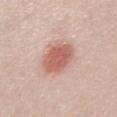Q: Was this lesion biopsied?
A: catalogued during a skin exam; not biopsied
Q: What is the imaging modality?
A: ~15 mm crop, total-body skin-cancer survey
Q: What did automated image analysis measure?
A: an area of roughly 11 mm² and a shape-asymmetry score of about 0.1 (0 = symmetric); roughly 13 lightness units darker than nearby skin and a lesion-to-skin contrast of about 8.5 (normalized; higher = more distinct); a border-irregularity index near 1.5/10, internal color variation of about 3 on a 0–10 scale, and radial color variation of about 1; an automated nevus-likeness rating near 100 out of 100 and lesion-presence confidence of about 100/100
Q: Where on the body is the lesion?
A: the chest
Q: Who is the patient?
A: female, aged 63 to 67
Q: Illumination type?
A: white-light illumination